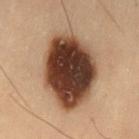biopsy status: no biopsy performed (imaged during a skin exam) | acquisition: total-body-photography crop, ~15 mm field of view | automated lesion analysis: an average lesion color of about L≈32 a*≈16 b*≈23 (CIELAB) and a lesion–skin lightness drop of about 21; a peripheral color-asymmetry measure near 2 | lighting: cross-polarized | diameter: ~8.5 mm (longest diameter) | location: the lower back | subject: male, approximately 55 years of age.